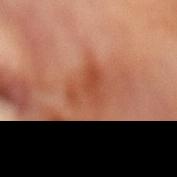{
  "biopsy_status": "not biopsied; imaged during a skin examination",
  "lighting": "cross-polarized",
  "image": {
    "source": "total-body photography crop",
    "field_of_view_mm": 15
  },
  "patient": {
    "sex": "female",
    "age_approx": 30
  },
  "lesion_size": {
    "long_diameter_mm_approx": 4.5
  },
  "site": "abdomen"
}